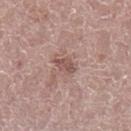  biopsy_status: not biopsied; imaged during a skin examination
  site: left lower leg
  patient:
    sex: male
    age_approx: 65
  image:
    source: total-body photography crop
    field_of_view_mm: 15
  lighting: white-light
  lesion_size:
    long_diameter_mm_approx: 2.5